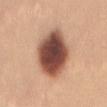No biopsy was performed on this lesion — it was imaged during a full skin examination and was not determined to be concerning. Located on the mid back. Cropped from a whole-body photographic skin survey; the tile spans about 15 mm. About 7 mm across. This is a white-light tile. A female subject, in their mid- to late 20s.